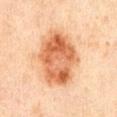The lesion was photographed on a routine skin check and not biopsied; there is no pathology result.
About 8 mm across.
The lesion is on the chest.
A male subject in their mid- to late 40s.
Captured under cross-polarized illumination.
A close-up tile cropped from a whole-body skin photograph, about 15 mm across.